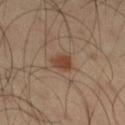Findings:
• workup — total-body-photography surveillance lesion; no biopsy
• subject — male, aged approximately 55
• imaging modality — total-body-photography crop, ~15 mm field of view
• location — the right thigh
• illumination — cross-polarized